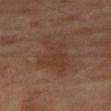Q: Was this lesion biopsied?
A: total-body-photography surveillance lesion; no biopsy
Q: What lighting was used for the tile?
A: cross-polarized illumination
Q: What did automated image analysis measure?
A: a mean CIELAB color near L≈36 a*≈18 b*≈25 and a lesion-to-skin contrast of about 5 (normalized; higher = more distinct); peripheral color asymmetry of about 1.5
Q: Who is the patient?
A: male, roughly 70 years of age
Q: What is the lesion's diameter?
A: ≈6 mm
Q: What is the anatomic site?
A: the right thigh
Q: What kind of image is this?
A: ~15 mm tile from a whole-body skin photo